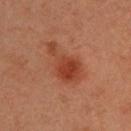The lesion's longest dimension is about 5.5 mm.
A 15 mm close-up extracted from a 3D total-body photography capture.
A male subject, in their mid- to late 30s.
Located on the upper back.
Automated tile analysis of the lesion measured a mean CIELAB color near L≈41 a*≈28 b*≈33, a lesion–skin lightness drop of about 9, and a lesion-to-skin contrast of about 7.5 (normalized; higher = more distinct). The software also gave internal color variation of about 4 on a 0–10 scale. The analysis additionally found a nevus-likeness score of about 100/100 and lesion-presence confidence of about 100/100.